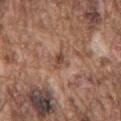Findings:
• workup · catalogued during a skin exam; not biopsied
• imaging modality · ~15 mm crop, total-body skin-cancer survey
• location · the mid back
• patient · male, aged 73 to 77